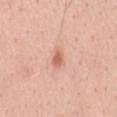Assessment: The lesion was tiled from a total-body skin photograph and was not biopsied. Context: The recorded lesion diameter is about 3 mm. The tile uses white-light illumination. Located on the back. The lesion-visualizer software estimated an area of roughly 3.5 mm², a shape eccentricity near 0.85, and two-axis asymmetry of about 0.25. And it measured a lesion color around L≈64 a*≈24 b*≈32 in CIELAB, roughly 10 lightness units darker than nearby skin, and a lesion-to-skin contrast of about 7 (normalized; higher = more distinct). A lesion tile, about 15 mm wide, cut from a 3D total-body photograph. A male subject about 55 years old.